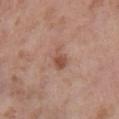Captured during whole-body skin photography for melanoma surveillance; the lesion was not biopsied. From the chest. The lesion-visualizer software estimated a footprint of about 4.5 mm², an eccentricity of roughly 0.85, and a shape-asymmetry score of about 0.3 (0 = symmetric). The software also gave about 9 CIELAB-L* units darker than the surrounding skin and a lesion-to-skin contrast of about 7 (normalized; higher = more distinct). The software also gave a border-irregularity index near 3.5/10, a within-lesion color-variation index near 2/10, and peripheral color asymmetry of about 0.5. Cropped from a total-body skin-imaging series; the visible field is about 15 mm. About 3 mm across. A male patient aged 53–57. Captured under white-light illumination.